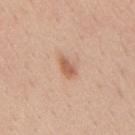  biopsy_status: not biopsied; imaged during a skin examination
  image:
    source: total-body photography crop
    field_of_view_mm: 15
  patient:
    sex: male
    age_approx: 30
  site: mid back
  lesion_size:
    long_diameter_mm_approx: 2.5
  lighting: white-light